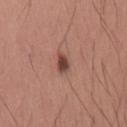This lesion was catalogued during total-body skin photography and was not selected for biopsy. A region of skin cropped from a whole-body photographic capture, roughly 15 mm wide. From the mid back. A male subject in their mid-30s. The lesion's longest dimension is about 2.5 mm. This is a white-light tile.